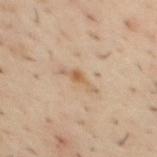Part of a total-body skin-imaging series; this lesion was reviewed on a skin check and was not flagged for biopsy.
A 15 mm crop from a total-body photograph taken for skin-cancer surveillance.
The lesion is on the back.
A male subject, aged around 40.